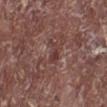Part of a total-body skin-imaging series; this lesion was reviewed on a skin check and was not flagged for biopsy. A 15 mm close-up extracted from a 3D total-body photography capture. Captured under white-light illumination. Longest diameter approximately 3 mm. On the leg. The subject is a male aged approximately 65. Automated image analysis of the tile measured a mean CIELAB color near L≈38 a*≈21 b*≈20, about 7 CIELAB-L* units darker than the surrounding skin, and a normalized lesion–skin contrast near 6.5. The software also gave border irregularity of about 4 on a 0–10 scale, a color-variation rating of about 1.5/10, and peripheral color asymmetry of about 0.5.